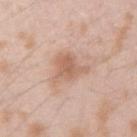Clinical impression: Recorded during total-body skin imaging; not selected for excision or biopsy. Acquisition and patient details: Imaged with white-light lighting. About 4.5 mm across. From the right upper arm. The subject is a male approximately 25 years of age. The lesion-visualizer software estimated an automated nevus-likeness rating near 10 out of 100 and a detector confidence of about 100 out of 100 that the crop contains a lesion. A 15 mm close-up extracted from a 3D total-body photography capture.